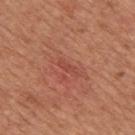Notes:
• workup — catalogued during a skin exam; not biopsied
• patient — male, roughly 65 years of age
• size — ~2.5 mm (longest diameter)
• image — ~15 mm tile from a whole-body skin photo
• illumination — white-light
• body site — the mid back
• image-analysis metrics — a shape-asymmetry score of about 0.3 (0 = symmetric); a classifier nevus-likeness of about 0/100 and a detector confidence of about 95 out of 100 that the crop contains a lesion